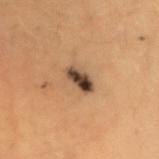The tile uses cross-polarized illumination. Approximately 3.5 mm at its widest. A male patient aged 38–42. On the right upper arm. A 15 mm close-up extracted from a 3D total-body photography capture.